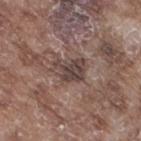follow-up: no biopsy performed (imaged during a skin exam) | lighting: white-light illumination | patient: male, roughly 75 years of age | diameter: ≈3.5 mm | TBP lesion metrics: a mean CIELAB color near L≈42 a*≈15 b*≈19, roughly 10 lightness units darker than nearby skin, and a normalized lesion–skin contrast near 8.5; a border-irregularity index near 3.5/10, a within-lesion color-variation index near 4.5/10, and a peripheral color-asymmetry measure near 1.5; a classifier nevus-likeness of about 0/100 and a lesion-detection confidence of about 80/100 | acquisition: 15 mm crop, total-body photography | location: the right thigh.